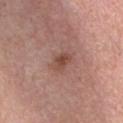  biopsy_status: not biopsied; imaged during a skin examination
  patient:
    sex: male
    age_approx: 45
  automated_metrics:
    area_mm2_approx: 4.5
    eccentricity: 0.8
    shape_asymmetry: 0.3
    lesion_detection_confidence_0_100: 100
  lighting: white-light
  image:
    source: total-body photography crop
    field_of_view_mm: 15
  lesion_size:
    long_diameter_mm_approx: 3.0
  site: chest